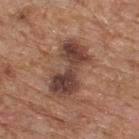workup = no biopsy performed (imaged during a skin exam)
subject = male, aged around 65
anatomic site = the back
tile lighting = white-light
imaging modality = ~15 mm tile from a whole-body skin photo
lesion diameter = about 6.5 mm